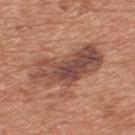No biopsy was performed on this lesion — it was imaged during a full skin examination and was not determined to be concerning.
The total-body-photography lesion software estimated a lesion area of about 24 mm², an outline eccentricity of about 0.9 (0 = round, 1 = elongated), and two-axis asymmetry of about 0.3. And it measured an average lesion color of about L≈48 a*≈22 b*≈27 (CIELAB).
The recorded lesion diameter is about 8.5 mm.
Located on the upper back.
A male patient aged approximately 65.
Cropped from a total-body skin-imaging series; the visible field is about 15 mm.